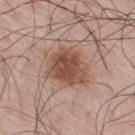Q: What lighting was used for the tile?
A: white-light
Q: How large is the lesion?
A: about 4.5 mm
Q: Patient demographics?
A: male, aged 68 to 72
Q: What is the imaging modality?
A: total-body-photography crop, ~15 mm field of view
Q: What did automated image analysis measure?
A: a footprint of about 15 mm², an eccentricity of roughly 0.45, and a symmetry-axis asymmetry near 0.2; border irregularity of about 2 on a 0–10 scale, a color-variation rating of about 4/10, and a peripheral color-asymmetry measure near 1
Q: What is the anatomic site?
A: the lower back